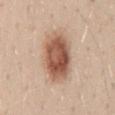The lesion was photographed on a routine skin check and not biopsied; there is no pathology result.
A female subject, aged 43 to 47.
Located on the mid back.
A 15 mm close-up extracted from a 3D total-body photography capture.
The total-body-photography lesion software estimated a lesion area of about 22 mm² and a shape-asymmetry score of about 0.1 (0 = symmetric). The software also gave a lesion–skin lightness drop of about 16 and a normalized lesion–skin contrast near 10. The software also gave border irregularity of about 1.5 on a 0–10 scale and radial color variation of about 2.
Imaged with white-light lighting.
The recorded lesion diameter is about 6.5 mm.